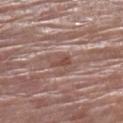The patient is a female in their 80s. The tile uses white-light illumination. A 15 mm crop from a total-body photograph taken for skin-cancer surveillance. The recorded lesion diameter is about 2.5 mm. Located on the left lower leg. Automated image analysis of the tile measured an average lesion color of about L≈48 a*≈20 b*≈24 (CIELAB) and a lesion–skin lightness drop of about 9. The software also gave a border-irregularity rating of about 4/10 and peripheral color asymmetry of about 0.5.